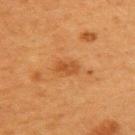follow-up — catalogued during a skin exam; not biopsied | location — the upper back | image — total-body-photography crop, ~15 mm field of view | patient — female, about 40 years old | tile lighting — cross-polarized illumination | diameter — ~2.5 mm (longest diameter).